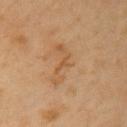Clinical impression:
Captured during whole-body skin photography for melanoma surveillance; the lesion was not biopsied.
Acquisition and patient details:
A lesion tile, about 15 mm wide, cut from a 3D total-body photograph. A female subject, aged approximately 50. Located on the upper back. An algorithmic analysis of the crop reported a footprint of about 2 mm² and an eccentricity of roughly 0.85. Imaged with cross-polarized lighting.